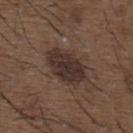The lesion was photographed on a routine skin check and not biopsied; there is no pathology result. On the upper back. Imaged with white-light lighting. The lesion's longest dimension is about 5.5 mm. A male patient roughly 50 years of age. A close-up tile cropped from a whole-body skin photograph, about 15 mm across.